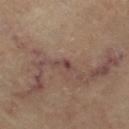follow-up — catalogued during a skin exam; not biopsied | size — ≈2.5 mm | image — total-body-photography crop, ~15 mm field of view | patient — female, in their mid-60s | body site — the leg | automated metrics — a border-irregularity index near 5/10, internal color variation of about 0 on a 0–10 scale, and peripheral color asymmetry of about 0; a classifier nevus-likeness of about 0/100 | lighting — cross-polarized.